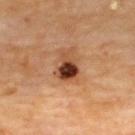No biopsy was performed on this lesion — it was imaged during a full skin examination and was not determined to be concerning.
The subject is a male aged 68 to 72.
The lesion is on the upper back.
The recorded lesion diameter is about 3.5 mm.
A roughly 15 mm field-of-view crop from a total-body skin photograph.